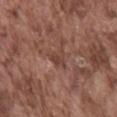site = the mid back | image source = 15 mm crop, total-body photography | automated lesion analysis = a lesion area of about 4 mm², a shape eccentricity near 0.65, and two-axis asymmetry of about 0.45; a border-irregularity rating of about 5/10; a lesion-detection confidence of about 65/100 | tile lighting = white-light | lesion size = ~3 mm (longest diameter) | subject = male, in their mid-70s.